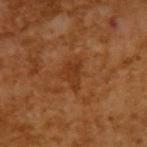Clinical impression: Imaged during a routine full-body skin examination; the lesion was not biopsied and no histopathology is available. Image and clinical context: A region of skin cropped from a whole-body photographic capture, roughly 15 mm wide. The recorded lesion diameter is about 3.5 mm. A male subject aged 63–67. The tile uses cross-polarized illumination.